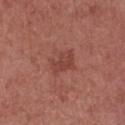Context:
On the chest. A male subject aged approximately 70. Automated image analysis of the tile measured an average lesion color of about L≈43 a*≈26 b*≈27 (CIELAB), about 7 CIELAB-L* units darker than the surrounding skin, and a normalized border contrast of about 5.5. And it measured a border-irregularity rating of about 4/10, a within-lesion color-variation index near 2/10, and a peripheral color-asymmetry measure near 1. And it measured an automated nevus-likeness rating near 0 out of 100 and a detector confidence of about 100 out of 100 that the crop contains a lesion. About 3.5 mm across. A close-up tile cropped from a whole-body skin photograph, about 15 mm across. Imaged with white-light lighting.